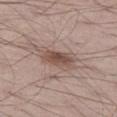Part of a total-body skin-imaging series; this lesion was reviewed on a skin check and was not flagged for biopsy. Cropped from a total-body skin-imaging series; the visible field is about 15 mm. A male patient, roughly 70 years of age. Located on the left thigh. The recorded lesion diameter is about 5 mm. The total-body-photography lesion software estimated a lesion color around L≈51 a*≈16 b*≈23 in CIELAB, about 10 CIELAB-L* units darker than the surrounding skin, and a normalized lesion–skin contrast near 7.5. And it measured an automated nevus-likeness rating near 85 out of 100 and lesion-presence confidence of about 100/100.